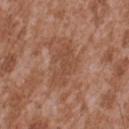The lesion was photographed on a routine skin check and not biopsied; there is no pathology result. From the upper back. A male patient, aged approximately 45. A 15 mm close-up tile from a total-body photography series done for melanoma screening.